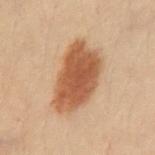A male patient, in their 30s.
A 15 mm close-up extracted from a 3D total-body photography capture.
From the abdomen.